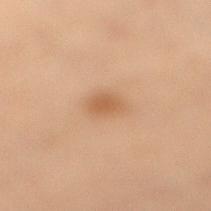notes: no biopsy performed (imaged during a skin exam)
patient: male, aged 53 to 57
image source: total-body-photography crop, ~15 mm field of view
location: the right lower leg
automated metrics: a mean CIELAB color near L≈48 a*≈17 b*≈30, roughly 7 lightness units darker than nearby skin, and a lesion-to-skin contrast of about 6 (normalized; higher = more distinct)
lighting: cross-polarized illumination